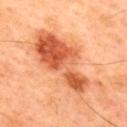  biopsy_status: not biopsied; imaged during a skin examination
  patient:
    sex: male
    age_approx: 45
  site: upper back
  image:
    source: total-body photography crop
    field_of_view_mm: 15
  automated_metrics:
    cielab_L: 58
    cielab_a: 30
    cielab_b: 40
    vs_skin_darker_L: 13.0
    vs_skin_contrast_norm: 8.0
    nevus_likeness_0_100: 30
    lesion_detection_confidence_0_100: 100
  lighting: cross-polarized
  lesion_size:
    long_diameter_mm_approx: 10.5